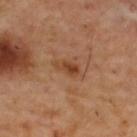The lesion was tiled from a total-body skin photograph and was not biopsied. About 3 mm across. The patient is a male in their mid-50s. This is a cross-polarized tile. An algorithmic analysis of the crop reported an average lesion color of about L≈41 a*≈23 b*≈33 (CIELAB), a lesion–skin lightness drop of about 9, and a normalized lesion–skin contrast near 7.5. And it measured a within-lesion color-variation index near 2/10 and peripheral color asymmetry of about 0.5. From the upper back. This image is a 15 mm lesion crop taken from a total-body photograph.